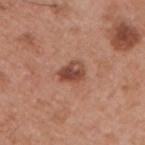notes: imaged on a skin check; not biopsied | location: the arm | patient: male, aged 53 to 57 | tile lighting: white-light | image: ~15 mm crop, total-body skin-cancer survey.